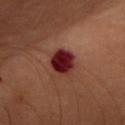Clinical impression:
The lesion was tiled from a total-body skin photograph and was not biopsied.
Image and clinical context:
A 15 mm crop from a total-body photograph taken for skin-cancer surveillance. The patient is a female aged 48 to 52. On the chest.